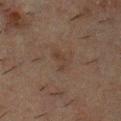Context:
The patient is a male aged around 50. Cropped from a whole-body photographic skin survey; the tile spans about 15 mm. Automated image analysis of the tile measured border irregularity of about 5.5 on a 0–10 scale and internal color variation of about 0 on a 0–10 scale. The lesion is on the chest.